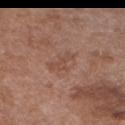Captured during whole-body skin photography for melanoma surveillance; the lesion was not biopsied.
A female subject roughly 80 years of age.
Captured under white-light illumination.
Cropped from a whole-body photographic skin survey; the tile spans about 15 mm.
The total-body-photography lesion software estimated an average lesion color of about L≈48 a*≈20 b*≈27 (CIELAB), a lesion–skin lightness drop of about 6, and a normalized border contrast of about 5. The software also gave a border-irregularity index near 5.5/10, a color-variation rating of about 0.5/10, and radial color variation of about 0.5. The software also gave a nevus-likeness score of about 0/100 and a detector confidence of about 100 out of 100 that the crop contains a lesion.
From the chest.
The recorded lesion diameter is about 3.5 mm.